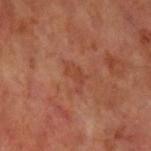Clinical impression: Imaged during a routine full-body skin examination; the lesion was not biopsied and no histopathology is available. Acquisition and patient details: From the left upper arm. A 15 mm close-up extracted from a 3D total-body photography capture. This is a cross-polarized tile. A male patient, about 65 years old.